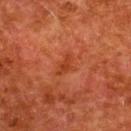| feature | finding |
|---|---|
| follow-up | no biopsy performed (imaged during a skin exam) |
| automated lesion analysis | a shape eccentricity near 0.8; about 6 CIELAB-L* units darker than the surrounding skin and a lesion-to-skin contrast of about 5.5 (normalized; higher = more distinct); a classifier nevus-likeness of about 0/100 and a detector confidence of about 100 out of 100 that the crop contains a lesion |
| location | the upper back |
| lighting | cross-polarized illumination |
| subject | female, about 50 years old |
| lesion size | about 3 mm |
| image | total-body-photography crop, ~15 mm field of view |